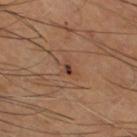Imaged during a routine full-body skin examination; the lesion was not biopsied and no histopathology is available. A lesion tile, about 15 mm wide, cut from a 3D total-body photograph. On the right thigh. The subject is a male approximately 70 years of age.